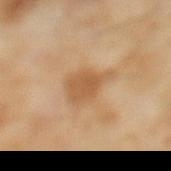Background:
A female patient aged 58–62. Imaged with cross-polarized lighting. A roughly 15 mm field-of-view crop from a total-body skin photograph. The lesion's longest dimension is about 5 mm. From the left lower leg. Automated image analysis of the tile measured an average lesion color of about L≈51 a*≈18 b*≈34 (CIELAB), about 8 CIELAB-L* units darker than the surrounding skin, and a lesion-to-skin contrast of about 6.5 (normalized; higher = more distinct). The software also gave a border-irregularity index near 3.5/10, a within-lesion color-variation index near 1.5/10, and a peripheral color-asymmetry measure near 0.5. The analysis additionally found a classifier nevus-likeness of about 0/100 and a lesion-detection confidence of about 100/100.